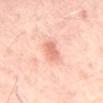Impression:
This lesion was catalogued during total-body skin photography and was not selected for biopsy.
Context:
Captured under cross-polarized illumination. Longest diameter approximately 4 mm. A 15 mm crop from a total-body photograph taken for skin-cancer surveillance. The lesion is located on the back. A patient aged approximately 55. Automated tile analysis of the lesion measured a footprint of about 5.5 mm², a shape eccentricity near 0.9, and two-axis asymmetry of about 0.25. It also reported a border-irregularity rating of about 3/10.